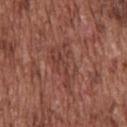Case summary:
- automated metrics · a footprint of about 14 mm²; a mean CIELAB color near L≈42 a*≈24 b*≈25, a lesion–skin lightness drop of about 6, and a normalized border contrast of about 5
- acquisition · ~15 mm tile from a whole-body skin photo
- lighting · white-light
- anatomic site · the back
- diameter · ≈7 mm
- subject · male, approximately 75 years of age